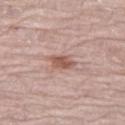workup: imaged on a skin check; not biopsied
body site: the leg
image source: ~15 mm crop, total-body skin-cancer survey
tile lighting: white-light illumination
lesion size: ≈3 mm
patient: female, aged around 65